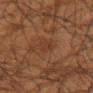{
  "biopsy_status": "not biopsied; imaged during a skin examination",
  "lighting": "cross-polarized",
  "lesion_size": {
    "long_diameter_mm_approx": 3.0
  },
  "image": {
    "source": "total-body photography crop",
    "field_of_view_mm": 15
  },
  "patient": {
    "sex": "male",
    "age_approx": 65
  },
  "site": "right upper arm",
  "automated_metrics": {
    "area_mm2_approx": 4.0,
    "eccentricity": 0.9,
    "shape_asymmetry": 0.25,
    "cielab_L": 29,
    "cielab_a": 18,
    "cielab_b": 26,
    "vs_skin_darker_L": 4.0,
    "vs_skin_contrast_norm": 5.0
  }
}